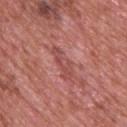Clinical impression: Imaged during a routine full-body skin examination; the lesion was not biopsied and no histopathology is available. Acquisition and patient details: Imaged with white-light lighting. A male patient aged 68 to 72. Located on the upper back. About 4.5 mm across. A roughly 15 mm field-of-view crop from a total-body skin photograph.